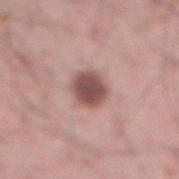Assessment:
Recorded during total-body skin imaging; not selected for excision or biopsy.
Acquisition and patient details:
A male subject roughly 65 years of age. The tile uses white-light illumination. Approximately 3.5 mm at its widest. The lesion is on the abdomen. A 15 mm crop from a total-body photograph taken for skin-cancer surveillance.